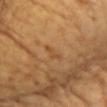The lesion is located on the chest. This image is a 15 mm lesion crop taken from a total-body photograph. A female subject aged 58 to 62.